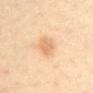Captured during whole-body skin photography for melanoma surveillance; the lesion was not biopsied. Approximately 4 mm at its widest. From the back. The lesion-visualizer software estimated a mean CIELAB color near L≈73 a*≈21 b*≈40, about 10 CIELAB-L* units darker than the surrounding skin, and a lesion-to-skin contrast of about 6 (normalized; higher = more distinct). The analysis additionally found a lesion-detection confidence of about 100/100. A region of skin cropped from a whole-body photographic capture, roughly 15 mm wide. A female patient in their mid-40s.